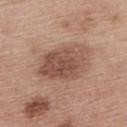| field | value |
|---|---|
| follow-up | no biopsy performed (imaged during a skin exam) |
| patient | female, aged 48 to 52 |
| acquisition | ~15 mm crop, total-body skin-cancer survey |
| anatomic site | the back |
| automated metrics | a lesion area of about 22 mm², an outline eccentricity of about 0.8 (0 = round, 1 = elongated), and two-axis asymmetry of about 0.2 |
| diameter | ~7 mm (longest diameter) |
| illumination | white-light illumination |